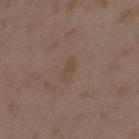<lesion>
  <automated_metrics>
    <area_mm2_approx>3.5</area_mm2_approx>
    <eccentricity>0.85</eccentricity>
    <shape_asymmetry>0.35</shape_asymmetry>
    <cielab_L>45</cielab_L>
    <cielab_a>14</cielab_a>
    <cielab_b>26</cielab_b>
    <vs_skin_darker_L>4.0</vs_skin_darker_L>
    <vs_skin_contrast_norm>5.0</vs_skin_contrast_norm>
    <nevus_likeness_0_100>0</nevus_likeness_0_100>
  </automated_metrics>
  <lesion_size>
    <long_diameter_mm_approx>3.0</long_diameter_mm_approx>
  </lesion_size>
  <image>
    <source>total-body photography crop</source>
    <field_of_view_mm>15</field_of_view_mm>
  </image>
  <site>left upper arm</site>
  <patient>
    <sex>female</sex>
    <age_approx>35</age_approx>
  </patient>
</lesion>